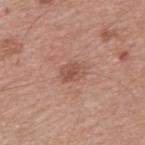Assessment:
No biopsy was performed on this lesion — it was imaged during a full skin examination and was not determined to be concerning.
Image and clinical context:
A male patient, aged 38–42. The lesion is on the upper back. This image is a 15 mm lesion crop taken from a total-body photograph.